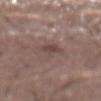Assessment:
This lesion was catalogued during total-body skin photography and was not selected for biopsy.
Image and clinical context:
A male patient aged 53–57. The lesion's longest dimension is about 4.5 mm. The tile uses white-light illumination. A 15 mm close-up tile from a total-body photography series done for melanoma screening. From the abdomen.